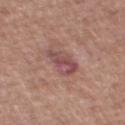  site: chest
  patient:
    sex: male
    age_approx: 65
  lesion_size:
    long_diameter_mm_approx: 4.5
  image:
    source: total-body photography crop
    field_of_view_mm: 15